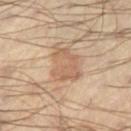Assessment:
No biopsy was performed on this lesion — it was imaged during a full skin examination and was not determined to be concerning.
Context:
This is a cross-polarized tile. The subject is a male approximately 45 years of age. Measured at roughly 4 mm in maximum diameter. On the leg. The total-body-photography lesion software estimated an outline eccentricity of about 0.6 (0 = round, 1 = elongated) and a shape-asymmetry score of about 0.3 (0 = symmetric). The analysis additionally found a mean CIELAB color near L≈58 a*≈18 b*≈31, about 8 CIELAB-L* units darker than the surrounding skin, and a normalized border contrast of about 5.5. The software also gave a classifier nevus-likeness of about 70/100. A lesion tile, about 15 mm wide, cut from a 3D total-body photograph.